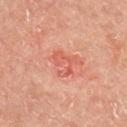No biopsy was performed on this lesion — it was imaged during a full skin examination and was not determined to be concerning. The tile uses cross-polarized illumination. A male patient aged 63–67. Measured at roughly 3.5 mm in maximum diameter. On the right upper arm. A close-up tile cropped from a whole-body skin photograph, about 15 mm across.